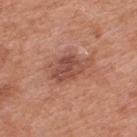{"biopsy_status": "not biopsied; imaged during a skin examination", "automated_metrics": {"area_mm2_approx": 12.0, "shape_asymmetry": 0.3, "cielab_L": 50, "cielab_a": 25, "cielab_b": 29, "vs_skin_darker_L": 10.0, "vs_skin_contrast_norm": 7.0, "border_irregularity_0_10": 3.5, "peripheral_color_asymmetry": 1.5, "nevus_likeness_0_100": 45, "lesion_detection_confidence_0_100": 100}, "lighting": "white-light", "image": {"source": "total-body photography crop", "field_of_view_mm": 15}, "site": "upper back", "patient": {"sex": "male", "age_approx": 70}, "lesion_size": {"long_diameter_mm_approx": 5.5}}